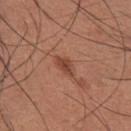Q: Is there a histopathology result?
A: catalogued during a skin exam; not biopsied
Q: Lesion location?
A: the chest
Q: What lighting was used for the tile?
A: white-light
Q: What is the imaging modality?
A: ~15 mm tile from a whole-body skin photo
Q: Who is the patient?
A: male, aged around 35
Q: What did automated image analysis measure?
A: an area of roughly 4 mm², an eccentricity of roughly 0.85, and two-axis asymmetry of about 0.25; a border-irregularity index near 2.5/10, a color-variation rating of about 1.5/10, and peripheral color asymmetry of about 0.5; an automated nevus-likeness rating near 55 out of 100